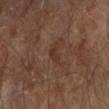The lesion was tiled from a total-body skin photograph and was not biopsied.
The lesion is located on the right forearm.
Longest diameter approximately 3 mm.
Cropped from a total-body skin-imaging series; the visible field is about 15 mm.
The subject is a male roughly 85 years of age or older.
This is a cross-polarized tile.